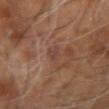Assessment:
The lesion was photographed on a routine skin check and not biopsied; there is no pathology result.
Clinical summary:
An algorithmic analysis of the crop reported a border-irregularity rating of about 4.5/10, a color-variation rating of about 0/10, and a peripheral color-asymmetry measure near 0. It also reported a classifier nevus-likeness of about 0/100 and lesion-presence confidence of about 95/100. The lesion's longest dimension is about 3.5 mm. This is a cross-polarized tile. A lesion tile, about 15 mm wide, cut from a 3D total-body photograph. From the right arm. The subject is a male aged 58–62.